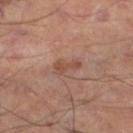<tbp_lesion>
<biopsy_status>not biopsied; imaged during a skin examination</biopsy_status>
<image>
  <source>total-body photography crop</source>
  <field_of_view_mm>15</field_of_view_mm>
</image>
<patient>
  <sex>male</sex>
  <age_approx>55</age_approx>
</patient>
<site>left lower leg</site>
</tbp_lesion>